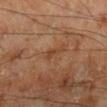Assessment:
No biopsy was performed on this lesion — it was imaged during a full skin examination and was not determined to be concerning.
Context:
The recorded lesion diameter is about 2.5 mm. A 15 mm close-up tile from a total-body photography series done for melanoma screening. From the right lower leg. The subject is a male aged 68 to 72. The tile uses cross-polarized illumination. The total-body-photography lesion software estimated an area of roughly 3 mm², an eccentricity of roughly 0.85, and a shape-asymmetry score of about 0.45 (0 = symmetric). The software also gave a mean CIELAB color near L≈42 a*≈21 b*≈33, about 6 CIELAB-L* units darker than the surrounding skin, and a normalized lesion–skin contrast near 5. It also reported a nevus-likeness score of about 0/100 and lesion-presence confidence of about 100/100.